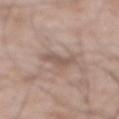Notes:
- notes · imaged on a skin check; not biopsied